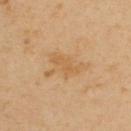automated_metrics:
  area_mm2_approx: 4.5
  eccentricity: 0.9
  vs_skin_darker_L: 6.0
  vs_skin_contrast_norm: 5.0
  border_irregularity_0_10: 5.5
  color_variation_0_10: 1.0
  peripheral_color_asymmetry: 0.0
image:
  source: total-body photography crop
  field_of_view_mm: 15
site: upper back
patient:
  sex: male
  age_approx: 55
lesion_size:
  long_diameter_mm_approx: 4.0
lighting: cross-polarized